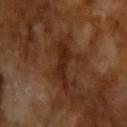| feature | finding |
|---|---|
| workup | imaged on a skin check; not biopsied |
| subject | male, in their mid- to late 60s |
| acquisition | ~15 mm tile from a whole-body skin photo |
| lesion size | ≈5.5 mm |
| lighting | cross-polarized |